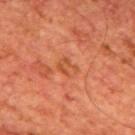{
  "biopsy_status": "not biopsied; imaged during a skin examination",
  "patient": {
    "sex": "male",
    "age_approx": 65
  },
  "image": {
    "source": "total-body photography crop",
    "field_of_view_mm": 15
  },
  "site": "mid back",
  "lesion_size": {
    "long_diameter_mm_approx": 2.5
  }
}